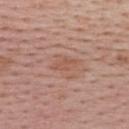workup = no biopsy performed (imaged during a skin exam)